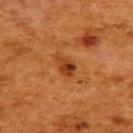| field | value |
|---|---|
| notes | imaged on a skin check; not biopsied |
| image source | ~15 mm tile from a whole-body skin photo |
| location | the upper back |
| illumination | cross-polarized |
| patient | female, aged approximately 50 |